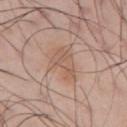illumination: white-light illumination; anatomic site: the left upper arm; image-analysis metrics: internal color variation of about 3 on a 0–10 scale and peripheral color asymmetry of about 1; subject: male, aged 43–47; lesion diameter: about 4 mm; image source: 15 mm crop, total-body photography.